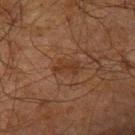Findings:
* lighting · cross-polarized
* subject · male, approximately 70 years of age
* lesion size · ≈3 mm
* body site · the left upper arm
* acquisition · ~15 mm crop, total-body skin-cancer survey